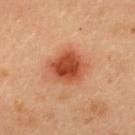Imaged during a routine full-body skin examination; the lesion was not biopsied and no histopathology is available. The subject is a female aged 33–37. Located on the upper back. This image is a 15 mm lesion crop taken from a total-body photograph. This is a cross-polarized tile. About 5 mm across.